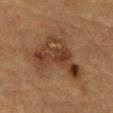<case>
  <biopsy_status>not biopsied; imaged during a skin examination</biopsy_status>
  <automated_metrics>
    <area_mm2_approx>21.0</area_mm2_approx>
    <eccentricity>0.7</eccentricity>
    <shape_asymmetry>0.6</shape_asymmetry>
    <cielab_L>33</cielab_L>
    <cielab_a>17</cielab_a>
    <cielab_b>27</cielab_b>
    <vs_skin_darker_L>9.0</vs_skin_darker_L>
    <vs_skin_contrast_norm>8.0</vs_skin_contrast_norm>
    <border_irregularity_0_10>6.5</border_irregularity_0_10>
    <color_variation_0_10>6.5</color_variation_0_10>
    <peripheral_color_asymmetry>2.0</peripheral_color_asymmetry>
    <nevus_likeness_0_100>0</nevus_likeness_0_100>
  </automated_metrics>
  <image>
    <source>total-body photography crop</source>
    <field_of_view_mm>15</field_of_view_mm>
  </image>
  <site>abdomen</site>
  <patient>
    <sex>male</sex>
    <age_approx>85+</age_approx>
  </patient>
</case>